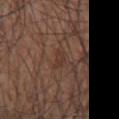The lesion is on the left upper arm. A male subject aged 58–62. This image is a 15 mm lesion crop taken from a total-body photograph.